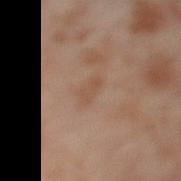Notes:
• follow-up: no biopsy performed (imaged during a skin exam)
• subject: female, aged 53 to 57
• lighting: cross-polarized illumination
• size: about 3 mm
• body site: the left thigh
• acquisition: ~15 mm crop, total-body skin-cancer survey
• automated metrics: a lesion area of about 3 mm², a shape eccentricity near 0.9, and a shape-asymmetry score of about 0.35 (0 = symmetric); a nevus-likeness score of about 0/100 and lesion-presence confidence of about 100/100